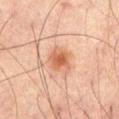<lesion>
<biopsy_status>not biopsied; imaged during a skin examination</biopsy_status>
<image>
  <source>total-body photography crop</source>
  <field_of_view_mm>15</field_of_view_mm>
</image>
<patient>
  <sex>male</sex>
  <age_approx>65</age_approx>
</patient>
<lighting>cross-polarized</lighting>
<lesion_size>
  <long_diameter_mm_approx>3.0</long_diameter_mm_approx>
</lesion_size>
<site>back</site>
</lesion>